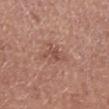Captured during whole-body skin photography for melanoma surveillance; the lesion was not biopsied. A 15 mm close-up tile from a total-body photography series done for melanoma screening. The subject is a male aged approximately 65. On the right lower leg.